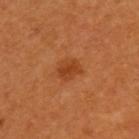The lesion was tiled from a total-body skin photograph and was not biopsied. On the upper back. This image is a 15 mm lesion crop taken from a total-body photograph. The subject is a male aged around 60. The lesion's longest dimension is about 2.5 mm. The tile uses cross-polarized illumination.